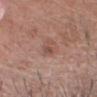The lesion was tiled from a total-body skin photograph and was not biopsied. Automated image analysis of the tile measured a lesion area of about 3 mm², a shape eccentricity near 0.85, and a symmetry-axis asymmetry near 0.35. And it measured a mean CIELAB color near L≈50 a*≈20 b*≈26 and a normalized border contrast of about 5.5. This image is a 15 mm lesion crop taken from a total-body photograph. A male subject, aged around 55. About 2.5 mm across. The tile uses white-light illumination. From the head or neck.